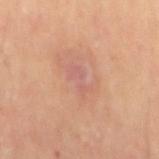No biopsy was performed on this lesion — it was imaged during a full skin examination and was not determined to be concerning.
Cropped from a total-body skin-imaging series; the visible field is about 15 mm.
The total-body-photography lesion software estimated a lesion color around L≈58 a*≈23 b*≈26 in CIELAB, roughly 6 lightness units darker than nearby skin, and a lesion-to-skin contrast of about 5 (normalized; higher = more distinct). It also reported a lesion-detection confidence of about 95/100.
A male subject, aged approximately 55.
From the mid back.